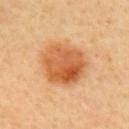This lesion was catalogued during total-body skin photography and was not selected for biopsy.
Automated image analysis of the tile measured a nevus-likeness score of about 100/100.
This is a cross-polarized tile.
Approximately 5.5 mm at its widest.
From the upper back.
A 15 mm crop from a total-body photograph taken for skin-cancer surveillance.
The subject is a female in their mid-30s.